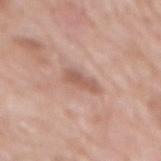Acquisition and patient details:
From the mid back. A 15 mm close-up extracted from a 3D total-body photography capture. The patient is a female roughly 75 years of age.